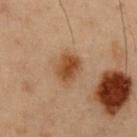{"biopsy_status": "not biopsied; imaged during a skin examination", "image": {"source": "total-body photography crop", "field_of_view_mm": 15}, "patient": {"sex": "male", "age_approx": 55}, "site": "chest"}